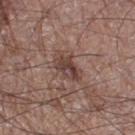Imaged during a routine full-body skin examination; the lesion was not biopsied and no histopathology is available. A close-up tile cropped from a whole-body skin photograph, about 15 mm across. Automated tile analysis of the lesion measured a border-irregularity rating of about 2.5/10, a within-lesion color-variation index near 5.5/10, and a peripheral color-asymmetry measure near 2. The subject is a male roughly 60 years of age. The lesion is located on the right thigh.